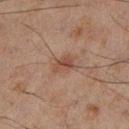Imaged during a routine full-body skin examination; the lesion was not biopsied and no histopathology is available. Located on the left lower leg. Cropped from a whole-body photographic skin survey; the tile spans about 15 mm. The patient is a male about 45 years old.